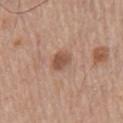Q: What is the imaging modality?
A: ~15 mm crop, total-body skin-cancer survey
Q: What is the anatomic site?
A: the chest
Q: What lighting was used for the tile?
A: white-light illumination
Q: How large is the lesion?
A: ~2.5 mm (longest diameter)
Q: Patient demographics?
A: male, in their mid-60s
Q: What did automated image analysis measure?
A: an automated nevus-likeness rating near 65 out of 100 and a lesion-detection confidence of about 100/100